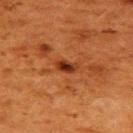notes=no biopsy performed (imaged during a skin exam) | acquisition=15 mm crop, total-body photography | location=the upper back | subject=female, aged 48 to 52.